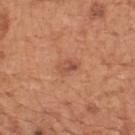follow-up: catalogued during a skin exam; not biopsied
image source: total-body-photography crop, ~15 mm field of view
size: ≈2.5 mm
patient: male, aged 63 to 67
site: the mid back
illumination: white-light illumination